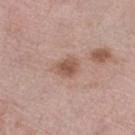notes = imaged on a skin check; not biopsied | image = 15 mm crop, total-body photography | subject = female, aged around 65 | automated metrics = a mean CIELAB color near L≈53 a*≈20 b*≈27; a detector confidence of about 100 out of 100 that the crop contains a lesion | body site = the leg | lesion diameter = ≈2.5 mm | tile lighting = white-light illumination.